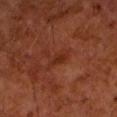Case summary:
• follow-up · catalogued during a skin exam; not biopsied
• subject · male, roughly 60 years of age
• TBP lesion metrics · a footprint of about 3.5 mm², an outline eccentricity of about 0.9 (0 = round, 1 = elongated), and a symmetry-axis asymmetry near 0.25; a lesion color around L≈28 a*≈25 b*≈29 in CIELAB, roughly 6 lightness units darker than nearby skin, and a normalized border contrast of about 6.5; a lesion-detection confidence of about 100/100
• anatomic site · the left forearm
• image · ~15 mm tile from a whole-body skin photo
• lighting · cross-polarized illumination